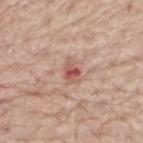  biopsy_status: not biopsied; imaged during a skin examination
  lighting: white-light
  lesion_size:
    long_diameter_mm_approx: 3.0
  image:
    source: total-body photography crop
    field_of_view_mm: 15
  patient:
    sex: male
    age_approx: 65
  site: back
  automated_metrics:
    area_mm2_approx: 4.5
    eccentricity: 0.75
    shape_asymmetry: 0.3
    cielab_L: 56
    cielab_a: 25
    cielab_b: 26
    vs_skin_darker_L: 11.0
    vs_skin_contrast_norm: 7.5
    nevus_likeness_0_100: 0
    lesion_detection_confidence_0_100: 100